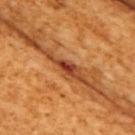The lesion was photographed on a routine skin check and not biopsied; there is no pathology result. Imaged with cross-polarized lighting. Automated image analysis of the tile measured a footprint of about 2.5 mm², an outline eccentricity of about 0.9 (0 = round, 1 = elongated), and a shape-asymmetry score of about 0.4 (0 = symmetric). The software also gave a lesion color around L≈43 a*≈35 b*≈39 in CIELAB and roughly 15 lightness units darker than nearby skin. It also reported a border-irregularity index near 3.5/10, internal color variation of about 2 on a 0–10 scale, and a peripheral color-asymmetry measure near 0. The recorded lesion diameter is about 2.5 mm. A female patient roughly 55 years of age. From the right upper arm. A 15 mm close-up tile from a total-body photography series done for melanoma screening.